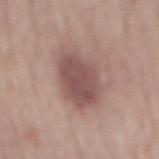Q: Is there a histopathology result?
A: total-body-photography surveillance lesion; no biopsy
Q: How large is the lesion?
A: ~6 mm (longest diameter)
Q: Where on the body is the lesion?
A: the mid back
Q: What is the imaging modality?
A: total-body-photography crop, ~15 mm field of view
Q: What are the patient's age and sex?
A: male, about 60 years old
Q: What lighting was used for the tile?
A: white-light illumination